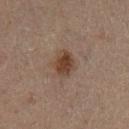Imaged during a routine full-body skin examination; the lesion was not biopsied and no histopathology is available. Automated image analysis of the tile measured a lesion area of about 6 mm². The software also gave a nevus-likeness score of about 95/100. On the left lower leg. A 15 mm crop from a total-body photograph taken for skin-cancer surveillance. About 3 mm across. The patient is a male roughly 70 years of age.